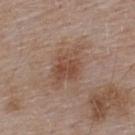<case>
<biopsy_status>not biopsied; imaged during a skin examination</biopsy_status>
<image>
  <source>total-body photography crop</source>
  <field_of_view_mm>15</field_of_view_mm>
</image>
<automated_metrics>
  <border_irregularity_0_10>4.0</border_irregularity_0_10>
  <color_variation_0_10>3.5</color_variation_0_10>
  <peripheral_color_asymmetry>1.5</peripheral_color_asymmetry>
</automated_metrics>
<patient>
  <sex>male</sex>
  <age_approx>55</age_approx>
</patient>
<lesion_size>
  <long_diameter_mm_approx>5.5</long_diameter_mm_approx>
</lesion_size>
<lighting>white-light</lighting>
<site>back</site>
</case>